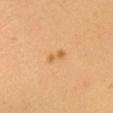Assessment: This lesion was catalogued during total-body skin photography and was not selected for biopsy. Image and clinical context: A female patient, aged 23 to 27. The lesion-visualizer software estimated roughly 9 lightness units darker than nearby skin and a normalized border contrast of about 6.5. And it measured a border-irregularity rating of about 4.5/10 and radial color variation of about 0. The software also gave a classifier nevus-likeness of about 60/100. The recorded lesion diameter is about 2.5 mm. Cropped from a whole-body photographic skin survey; the tile spans about 15 mm. Imaged with cross-polarized lighting. From the head or neck.